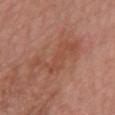No biopsy was performed on this lesion — it was imaged during a full skin examination and was not determined to be concerning.